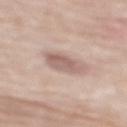From the mid back. A 15 mm close-up tile from a total-body photography series done for melanoma screening. Imaged with white-light lighting. Automated image analysis of the tile measured a lesion area of about 7.5 mm², a shape eccentricity near 0.7, and a shape-asymmetry score of about 0.2 (0 = symmetric). The software also gave radial color variation of about 1.5. A male patient, aged 78 to 82.